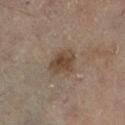Case summary:
* notes — total-body-photography surveillance lesion; no biopsy
* subject — female, approximately 60 years of age
* site — the leg
* diameter — ≈4 mm
* lighting — cross-polarized illumination
* automated metrics — a shape eccentricity near 0.7 and two-axis asymmetry of about 0.25; a nevus-likeness score of about 80/100 and lesion-presence confidence of about 100/100
* image — ~15 mm crop, total-body skin-cancer survey